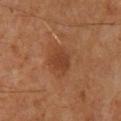Q: Was a biopsy performed?
A: no biopsy performed (imaged during a skin exam)
Q: Who is the patient?
A: male, aged approximately 60
Q: What lighting was used for the tile?
A: cross-polarized illumination
Q: What is the anatomic site?
A: the upper back
Q: What kind of image is this?
A: total-body-photography crop, ~15 mm field of view
Q: Lesion size?
A: ~3 mm (longest diameter)